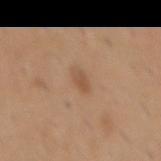workup — no biopsy performed (imaged during a skin exam) | diameter — about 2.5 mm | site — the back | automated metrics — a lesion area of about 3.5 mm², an outline eccentricity of about 0.8 (0 = round, 1 = elongated), and a symmetry-axis asymmetry near 0.25; about 8 CIELAB-L* units darker than the surrounding skin and a normalized lesion–skin contrast near 5.5; border irregularity of about 2 on a 0–10 scale, a color-variation rating of about 2/10, and peripheral color asymmetry of about 0.5; a nevus-likeness score of about 70/100 and a lesion-detection confidence of about 100/100 | subject — male, roughly 40 years of age | lighting — white-light illumination | image source — 15 mm crop, total-body photography.